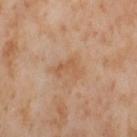Assessment:
The lesion was photographed on a routine skin check and not biopsied; there is no pathology result.
Image and clinical context:
A 15 mm close-up extracted from a 3D total-body photography capture. The lesion is located on the left thigh. Imaged with cross-polarized lighting. Automated tile analysis of the lesion measured a footprint of about 5.5 mm², an eccentricity of roughly 0.8, and two-axis asymmetry of about 0.5. And it measured border irregularity of about 5.5 on a 0–10 scale, a within-lesion color-variation index near 2/10, and a peripheral color-asymmetry measure near 1. The subject is a female in their mid- to late 50s.